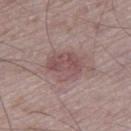Cropped from a whole-body photographic skin survey; the tile spans about 15 mm. The lesion is located on the leg. This is a white-light tile. A male subject, aged 73–77. The recorded lesion diameter is about 4 mm.